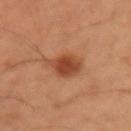Clinical impression:
Captured during whole-body skin photography for melanoma surveillance; the lesion was not biopsied.
Image and clinical context:
An algorithmic analysis of the crop reported a footprint of about 8 mm² and an eccentricity of roughly 0.6. It also reported a lesion color around L≈44 a*≈26 b*≈34 in CIELAB, about 11 CIELAB-L* units darker than the surrounding skin, and a lesion-to-skin contrast of about 8.5 (normalized; higher = more distinct). From the arm. A 15 mm close-up tile from a total-body photography series done for melanoma screening. Approximately 3.5 mm at its widest. The subject is a male in their mid- to late 50s.